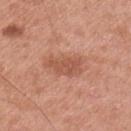anatomic site = the left upper arm | acquisition = ~15 mm tile from a whole-body skin photo | lesion diameter = ≈4.5 mm | subject = male, aged 53 to 57 | automated metrics = an area of roughly 9 mm², a shape eccentricity near 0.85, and a symmetry-axis asymmetry near 0.25; a lesion color around L≈55 a*≈25 b*≈31 in CIELAB and a lesion–skin lightness drop of about 9; a border-irregularity index near 3/10 and peripheral color asymmetry of about 1; a detector confidence of about 100 out of 100 that the crop contains a lesion | illumination = white-light illumination.